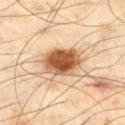Part of a total-body skin-imaging series; this lesion was reviewed on a skin check and was not flagged for biopsy. An algorithmic analysis of the crop reported a classifier nevus-likeness of about 100/100 and a detector confidence of about 100 out of 100 that the crop contains a lesion. A 15 mm crop from a total-body photograph taken for skin-cancer surveillance. A male patient, aged approximately 45. From the left thigh. The lesion's longest dimension is about 4.5 mm. Captured under cross-polarized illumination.